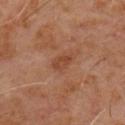| field | value |
|---|---|
| workup | total-body-photography surveillance lesion; no biopsy |
| patient | male, aged approximately 60 |
| illumination | cross-polarized |
| anatomic site | the chest |
| acquisition | ~15 mm crop, total-body skin-cancer survey |
| diameter | about 3 mm |